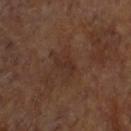biopsy status: catalogued during a skin exam; not biopsied
lesion diameter: ~2.5 mm (longest diameter)
site: the head or neck
patient: male, in their 60s
image: ~15 mm crop, total-body skin-cancer survey
TBP lesion metrics: a lesion area of about 2.5 mm², a shape eccentricity near 0.9, and a shape-asymmetry score of about 0.45 (0 = symmetric); a mean CIELAB color near L≈27 a*≈18 b*≈22 and about 5 CIELAB-L* units darker than the surrounding skin; a border-irregularity index near 5.5/10 and peripheral color asymmetry of about 0